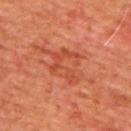Assessment:
This lesion was catalogued during total-body skin photography and was not selected for biopsy.
Background:
Measured at roughly 4 mm in maximum diameter. On the upper back. Cropped from a whole-body photographic skin survey; the tile spans about 15 mm. Automated image analysis of the tile measured lesion-presence confidence of about 100/100. Captured under cross-polarized illumination. A male patient about 65 years old.